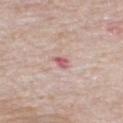Impression: Captured during whole-body skin photography for melanoma surveillance; the lesion was not biopsied. Background: The subject is a male in their mid-70s. A 15 mm close-up extracted from a 3D total-body photography capture. The lesion is located on the mid back. The lesion-visualizer software estimated a lesion area of about 3.5 mm², an outline eccentricity of about 0.75 (0 = round, 1 = elongated), and a symmetry-axis asymmetry near 0.25. The software also gave a mean CIELAB color near L≈61 a*≈24 b*≈20, about 10 CIELAB-L* units darker than the surrounding skin, and a lesion-to-skin contrast of about 6.5 (normalized; higher = more distinct). The analysis additionally found a border-irregularity index near 2.5/10, a within-lesion color-variation index near 4/10, and radial color variation of about 1. The analysis additionally found an automated nevus-likeness rating near 0 out of 100 and a lesion-detection confidence of about 100/100.